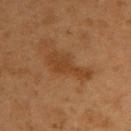* image — total-body-photography crop, ~15 mm field of view
* location — the upper back
* subject — male, roughly 55 years of age